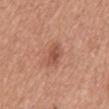Imaged during a routine full-body skin examination; the lesion was not biopsied and no histopathology is available. The patient is a female aged approximately 55. A roughly 15 mm field-of-view crop from a total-body skin photograph. The tile uses white-light illumination. An algorithmic analysis of the crop reported an area of roughly 5 mm², an outline eccentricity of about 0.65 (0 = round, 1 = elongated), and two-axis asymmetry of about 0.3. Located on the mid back. The lesion's longest dimension is about 3 mm.